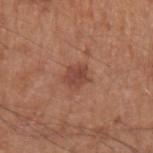Q: Is there a histopathology result?
A: no biopsy performed (imaged during a skin exam)
Q: What lighting was used for the tile?
A: white-light illumination
Q: How was this image acquired?
A: total-body-photography crop, ~15 mm field of view
Q: Where on the body is the lesion?
A: the left upper arm
Q: How large is the lesion?
A: ≈3 mm
Q: Patient demographics?
A: male, aged around 65